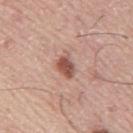Clinical impression:
Part of a total-body skin-imaging series; this lesion was reviewed on a skin check and was not flagged for biopsy.
Acquisition and patient details:
Automated image analysis of the tile measured a shape eccentricity near 0.75 and two-axis asymmetry of about 0.2. The analysis additionally found a lesion color around L≈53 a*≈23 b*≈27 in CIELAB, roughly 14 lightness units darker than nearby skin, and a normalized border contrast of about 9. From the mid back. Measured at roughly 3 mm in maximum diameter. The patient is a male aged approximately 75. Captured under white-light illumination. Cropped from a total-body skin-imaging series; the visible field is about 15 mm.